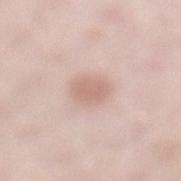• follow-up: catalogued during a skin exam; not biopsied
• image-analysis metrics: a border-irregularity rating of about 1.5/10 and internal color variation of about 1.5 on a 0–10 scale
• lighting: white-light illumination
• image: ~15 mm tile from a whole-body skin photo
• anatomic site: the left lower leg
• size: ≈2.5 mm
• patient: male, in their 50s